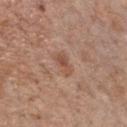This lesion was catalogued during total-body skin photography and was not selected for biopsy.
The tile uses white-light illumination.
The lesion-visualizer software estimated a lesion area of about 4 mm². And it measured an average lesion color of about L≈51 a*≈21 b*≈29 (CIELAB). And it measured a classifier nevus-likeness of about 0/100.
Located on the chest.
A male patient, aged around 70.
About 3 mm across.
A close-up tile cropped from a whole-body skin photograph, about 15 mm across.